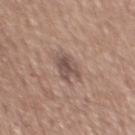* notes · catalogued during a skin exam; not biopsied
* diameter · ~3.5 mm (longest diameter)
* anatomic site · the mid back
* patient · male, in their mid-60s
* lighting · white-light
* image source · 15 mm crop, total-body photography
* automated lesion analysis · a lesion area of about 6.5 mm²; an average lesion color of about L≈51 a*≈16 b*≈22 (CIELAB) and a normalized lesion–skin contrast near 7.5; a border-irregularity index near 3/10, a within-lesion color-variation index near 4/10, and a peripheral color-asymmetry measure near 1.5; a classifier nevus-likeness of about 0/100 and a lesion-detection confidence of about 100/100